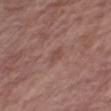workup=no biopsy performed (imaged during a skin exam) | site=the right thigh | acquisition=~15 mm crop, total-body skin-cancer survey | subject=male, in their 80s | TBP lesion metrics=a lesion area of about 3.5 mm², a shape eccentricity near 0.85, and a symmetry-axis asymmetry near 0.3; about 6 CIELAB-L* units darker than the surrounding skin and a normalized lesion–skin contrast near 4.5; a border-irregularity index near 3/10, a color-variation rating of about 1/10, and peripheral color asymmetry of about 0.5; a nevus-likeness score of about 0/100 and a lesion-detection confidence of about 100/100 | lighting=white-light.